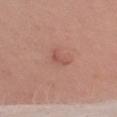The lesion is on the left upper arm. Captured under white-light illumination. Longest diameter approximately 2.5 mm. The lesion-visualizer software estimated a mean CIELAB color near L≈53 a*≈24 b*≈27. A close-up tile cropped from a whole-body skin photograph, about 15 mm across. The patient is a male aged around 55.